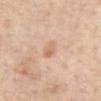| field | value |
|---|---|
| biopsy status | total-body-photography surveillance lesion; no biopsy |
| lesion size | about 2.5 mm |
| automated lesion analysis | an area of roughly 3.5 mm² and a shape-asymmetry score of about 0.25 (0 = symmetric); an average lesion color of about L≈67 a*≈18 b*≈33 (CIELAB), roughly 8 lightness units darker than nearby skin, and a lesion-to-skin contrast of about 5.5 (normalized; higher = more distinct); an automated nevus-likeness rating near 5 out of 100 |
| image | 15 mm crop, total-body photography |
| lighting | white-light illumination |
| location | the front of the torso |
| patient | female, aged around 65 |